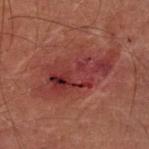Clinical impression: The lesion was tiled from a total-body skin photograph and was not biopsied. Background: A 15 mm close-up tile from a total-body photography series done for melanoma screening. The lesion is on the left forearm. A male subject, roughly 70 years of age. Imaged with cross-polarized lighting.